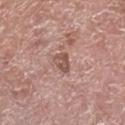| field | value |
|---|---|
| workup | no biopsy performed (imaged during a skin exam) |
| patient | male, aged 73 to 77 |
| location | the leg |
| acquisition | 15 mm crop, total-body photography |